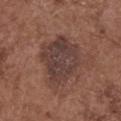- lighting: white-light
- automated lesion analysis: an area of roughly 24 mm², an eccentricity of roughly 0.6, and a symmetry-axis asymmetry near 0.25; a border-irregularity rating of about 3/10, internal color variation of about 4 on a 0–10 scale, and peripheral color asymmetry of about 1.5; lesion-presence confidence of about 100/100
- patient: female, aged 73–77
- site: the upper back
- size: ~6 mm (longest diameter)
- image: ~15 mm tile from a whole-body skin photo